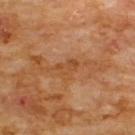Case summary:
- workup — imaged on a skin check; not biopsied
- lighting — cross-polarized
- image — ~15 mm crop, total-body skin-cancer survey
- lesion diameter — ~2.5 mm (longest diameter)
- anatomic site — the chest
- patient — male, in their 60s